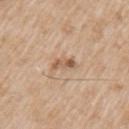notes = no biopsy performed (imaged during a skin exam).